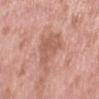The lesion was photographed on a routine skin check and not biopsied; there is no pathology result. Imaged with white-light lighting. A male patient approximately 55 years of age. Automated image analysis of the tile measured a classifier nevus-likeness of about 0/100 and a detector confidence of about 100 out of 100 that the crop contains a lesion. A 15 mm close-up tile from a total-body photography series done for melanoma screening. About 6 mm across. Located on the left forearm.